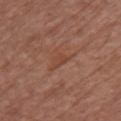Q: Was a biopsy performed?
A: total-body-photography surveillance lesion; no biopsy
Q: Lesion location?
A: the chest
Q: What did automated image analysis measure?
A: a shape eccentricity near 0.8 and two-axis asymmetry of about 0.4; border irregularity of about 4 on a 0–10 scale and radial color variation of about 0; a classifier nevus-likeness of about 0/100
Q: Patient demographics?
A: female, in their mid- to late 70s
Q: What is the lesion's diameter?
A: ~2.5 mm (longest diameter)
Q: What lighting was used for the tile?
A: white-light
Q: What is the imaging modality?
A: ~15 mm tile from a whole-body skin photo